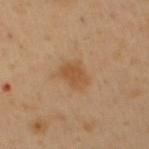The lesion was tiled from a total-body skin photograph and was not biopsied. The lesion is on the abdomen. The total-body-photography lesion software estimated a footprint of about 5.5 mm², an eccentricity of roughly 0.75, and a symmetry-axis asymmetry near 0.25. The analysis additionally found an average lesion color of about L≈50 a*≈20 b*≈36 (CIELAB), roughly 8 lightness units darker than nearby skin, and a normalized border contrast of about 7. The analysis additionally found border irregularity of about 2.5 on a 0–10 scale, internal color variation of about 1.5 on a 0–10 scale, and radial color variation of about 0.5. Captured under cross-polarized illumination. A 15 mm close-up tile from a total-body photography series done for melanoma screening. A male patient aged around 50. The lesion's longest dimension is about 3 mm.